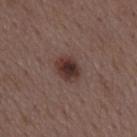Q: Was this lesion biopsied?
A: total-body-photography surveillance lesion; no biopsy
Q: Illumination type?
A: white-light illumination
Q: Lesion size?
A: ~3.5 mm (longest diameter)
Q: What did automated image analysis measure?
A: a mean CIELAB color near L≈33 a*≈18 b*≈20, a lesion–skin lightness drop of about 12, and a normalized border contrast of about 10.5
Q: What is the anatomic site?
A: the mid back
Q: How was this image acquired?
A: ~15 mm tile from a whole-body skin photo
Q: Patient demographics?
A: male, approximately 50 years of age